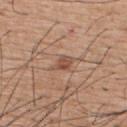The recorded lesion diameter is about 2.5 mm.
An algorithmic analysis of the crop reported a footprint of about 4 mm² and an outline eccentricity of about 0.7 (0 = round, 1 = elongated). The analysis additionally found a classifier nevus-likeness of about 80/100.
A 15 mm crop from a total-body photograph taken for skin-cancer surveillance.
A male patient, aged 53–57.
On the upper back.